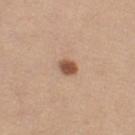Assessment: Imaged during a routine full-body skin examination; the lesion was not biopsied and no histopathology is available. Background: Approximately 2 mm at its widest. On the right thigh. A female patient, approximately 60 years of age. Captured under white-light illumination. A region of skin cropped from a whole-body photographic capture, roughly 15 mm wide.